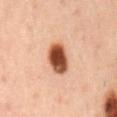Acquisition and patient details:
The total-body-photography lesion software estimated a lesion area of about 9.5 mm², an eccentricity of roughly 0.75, and a shape-asymmetry score of about 0.15 (0 = symmetric). It also reported an average lesion color of about L≈50 a*≈26 b*≈34 (CIELAB), roughly 23 lightness units darker than nearby skin, and a normalized lesion–skin contrast near 14.5. Imaged with cross-polarized lighting. The lesion's longest dimension is about 4 mm. A 15 mm close-up tile from a total-body photography series done for melanoma screening. The subject is a male about 55 years old. On the back.